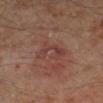{"biopsy_status": "not biopsied; imaged during a skin examination", "site": "leg", "automated_metrics": {"area_mm2_approx": 6.5, "eccentricity": 0.7}, "lesion_size": {"long_diameter_mm_approx": 3.5}, "patient": {"sex": "male", "age_approx": 50}, "image": {"source": "total-body photography crop", "field_of_view_mm": 15}}